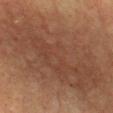Q: Is there a histopathology result?
A: no biopsy performed (imaged during a skin exam)
Q: What is the lesion's diameter?
A: about 13 mm
Q: Illumination type?
A: cross-polarized illumination
Q: What kind of image is this?
A: ~15 mm crop, total-body skin-cancer survey
Q: Lesion location?
A: the chest
Q: What are the patient's age and sex?
A: male, in their 60s